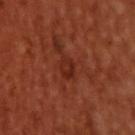Clinical impression: The lesion was photographed on a routine skin check and not biopsied; there is no pathology result. Background: Longest diameter approximately 3 mm. A male subject, roughly 50 years of age. From the upper back. This image is a 15 mm lesion crop taken from a total-body photograph. Captured under cross-polarized illumination.